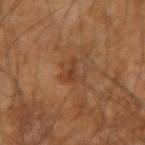notes = catalogued during a skin exam; not biopsied | lesion diameter = ~3 mm (longest diameter) | image = ~15 mm crop, total-body skin-cancer survey | patient = male, roughly 55 years of age | body site = the arm | illumination = cross-polarized | image-analysis metrics = about 7 CIELAB-L* units darker than the surrounding skin and a normalized border contrast of about 6; a within-lesion color-variation index near 2/10 and a peripheral color-asymmetry measure near 0.5; an automated nevus-likeness rating near 0 out of 100 and a lesion-detection confidence of about 100/100.